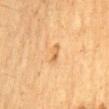Q: Was this lesion biopsied?
A: catalogued during a skin exam; not biopsied
Q: How was this image acquired?
A: ~15 mm crop, total-body skin-cancer survey
Q: Illumination type?
A: cross-polarized
Q: What is the anatomic site?
A: the mid back
Q: Lesion size?
A: ≈3 mm
Q: Patient demographics?
A: male, aged 58–62